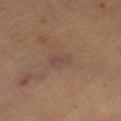- workup — catalogued during a skin exam; not biopsied
- patient — aged around 60
- imaging modality — 15 mm crop, total-body photography
- body site — the left thigh
- illumination — cross-polarized